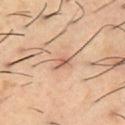Q: Was this lesion biopsied?
A: imaged on a skin check; not biopsied
Q: Lesion location?
A: the front of the torso
Q: What did automated image analysis measure?
A: a symmetry-axis asymmetry near 0.3; an average lesion color of about L≈59 a*≈20 b*≈31 (CIELAB), about 10 CIELAB-L* units darker than the surrounding skin, and a normalized lesion–skin contrast near 6.5; a border-irregularity rating of about 3/10, a within-lesion color-variation index near 3/10, and a peripheral color-asymmetry measure near 1
Q: What is the lesion's diameter?
A: about 2.5 mm
Q: What kind of image is this?
A: ~15 mm crop, total-body skin-cancer survey
Q: Patient demographics?
A: male, aged 53 to 57
Q: Illumination type?
A: cross-polarized illumination